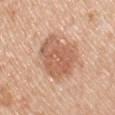Part of a total-body skin-imaging series; this lesion was reviewed on a skin check and was not flagged for biopsy. Located on the upper back. Imaged with white-light lighting. Longest diameter approximately 6.5 mm. A male subject, aged approximately 50. Automated tile analysis of the lesion measured a footprint of about 21 mm², an eccentricity of roughly 0.75, and two-axis asymmetry of about 0.25. And it measured a normalized border contrast of about 7. The analysis additionally found a within-lesion color-variation index near 3.5/10 and radial color variation of about 1.5. A region of skin cropped from a whole-body photographic capture, roughly 15 mm wide.